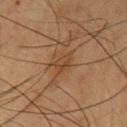Impression: The lesion was photographed on a routine skin check and not biopsied; there is no pathology result. Context: The recorded lesion diameter is about 2.5 mm. A male patient, in their mid- to late 50s. The lesion-visualizer software estimated an area of roughly 3.5 mm², a shape eccentricity near 0.7, and two-axis asymmetry of about 0.3. It also reported a border-irregularity rating of about 3/10, a color-variation rating of about 2.5/10, and peripheral color asymmetry of about 1. The software also gave an automated nevus-likeness rating near 0 out of 100 and lesion-presence confidence of about 65/100. Captured under cross-polarized illumination. A lesion tile, about 15 mm wide, cut from a 3D total-body photograph. From the right upper arm.